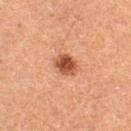Q: Was a biopsy performed?
A: total-body-photography surveillance lesion; no biopsy
Q: Where on the body is the lesion?
A: the left thigh
Q: How large is the lesion?
A: about 3 mm
Q: What is the imaging modality?
A: ~15 mm crop, total-body skin-cancer survey
Q: What lighting was used for the tile?
A: cross-polarized
Q: Who is the patient?
A: female, roughly 60 years of age